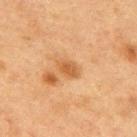<lesion>
<biopsy_status>not biopsied; imaged during a skin examination</biopsy_status>
<lighting>cross-polarized</lighting>
<image>
  <source>total-body photography crop</source>
  <field_of_view_mm>15</field_of_view_mm>
</image>
<patient>
  <sex>male</sex>
  <age_approx>75</age_approx>
</patient>
<automated_metrics>
  <eccentricity>0.75</eccentricity>
  <shape_asymmetry>0.15</shape_asymmetry>
  <cielab_L>47</cielab_L>
  <cielab_a>20</cielab_a>
  <cielab_b>36</cielab_b>
  <vs_skin_darker_L>8.0</vs_skin_darker_L>
  <vs_skin_contrast_norm>7.0</vs_skin_contrast_norm>
  <border_irregularity_0_10>1.5</border_irregularity_0_10>
  <color_variation_0_10>1.5</color_variation_0_10>
  <nevus_likeness_0_100>10</nevus_likeness_0_100>
</automated_metrics>
<lesion_size>
  <long_diameter_mm_approx>3.0</long_diameter_mm_approx>
</lesion_size>
<site>mid back</site>
</lesion>